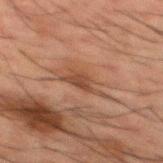Case summary:
* notes — catalogued during a skin exam; not biopsied
* location — the back
* image source — ~15 mm crop, total-body skin-cancer survey
* patient — male, approximately 50 years of age